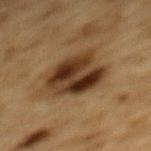The subject is a male in their mid- to late 80s or older.
From the mid back.
A region of skin cropped from a whole-body photographic capture, roughly 15 mm wide.